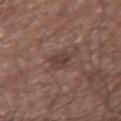  biopsy_status: not biopsied; imaged during a skin examination
  lighting: white-light
  image:
    source: total-body photography crop
    field_of_view_mm: 15
  lesion_size:
    long_diameter_mm_approx: 3.0
  site: arm
  patient:
    sex: male
    age_approx: 65
  automated_metrics:
    area_mm2_approx: 4.0
    eccentricity: 0.75
    shape_asymmetry: 0.3
    cielab_L: 39
    cielab_a: 17
    cielab_b: 20
    vs_skin_darker_L: 8.0
    vs_skin_contrast_norm: 6.5
    border_irregularity_0_10: 2.5
    color_variation_0_10: 2.5
    peripheral_color_asymmetry: 1.0